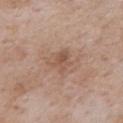Q: Where on the body is the lesion?
A: the back
Q: Patient demographics?
A: male, approximately 75 years of age
Q: Illumination type?
A: white-light
Q: What kind of image is this?
A: total-body-photography crop, ~15 mm field of view
Q: What did automated image analysis measure?
A: an area of roughly 4.5 mm² and two-axis asymmetry of about 0.4; about 8 CIELAB-L* units darker than the surrounding skin and a normalized lesion–skin contrast near 5.5; a border-irregularity index near 4/10 and peripheral color asymmetry of about 1
Q: How large is the lesion?
A: ~3 mm (longest diameter)